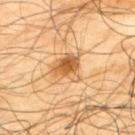Q: Is there a histopathology result?
A: imaged on a skin check; not biopsied
Q: Patient demographics?
A: male, approximately 65 years of age
Q: What lighting was used for the tile?
A: cross-polarized illumination
Q: What is the anatomic site?
A: the upper back
Q: How large is the lesion?
A: about 3.5 mm
Q: What is the imaging modality?
A: 15 mm crop, total-body photography